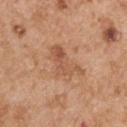biopsy status = no biopsy performed (imaged during a skin exam)
anatomic site = the right upper arm
subject = male, approximately 55 years of age
lighting = white-light
image source = total-body-photography crop, ~15 mm field of view
TBP lesion metrics = an average lesion color of about L≈55 a*≈23 b*≈34 (CIELAB) and roughly 8 lightness units darker than nearby skin; a nevus-likeness score of about 0/100 and a lesion-detection confidence of about 100/100